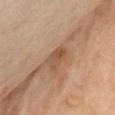Imaged during a routine full-body skin examination; the lesion was not biopsied and no histopathology is available.
Captured under cross-polarized illumination.
A female patient, aged 58 to 62.
The lesion is located on the chest.
Automated image analysis of the tile measured an automated nevus-likeness rating near 0 out of 100 and a detector confidence of about 100 out of 100 that the crop contains a lesion.
This image is a 15 mm lesion crop taken from a total-body photograph.